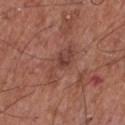Clinical summary: A 15 mm close-up tile from a total-body photography series done for melanoma screening. The recorded lesion diameter is about 5.5 mm. The patient is a male aged 73–77. The tile uses white-light illumination. The lesion is located on the chest.